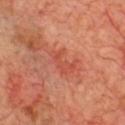{
  "biopsy_status": "not biopsied; imaged during a skin examination",
  "image": {
    "source": "total-body photography crop",
    "field_of_view_mm": 15
  },
  "patient": {
    "sex": "male",
    "age_approx": 70
  },
  "site": "chest"
}